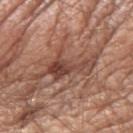follow-up = catalogued during a skin exam; not biopsied | illumination = white-light | patient = male, aged 63 to 67 | imaging modality = total-body-photography crop, ~15 mm field of view | location = the right upper arm.